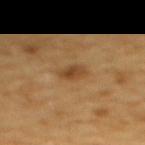patient: female, roughly 55 years of age | location: the upper back | acquisition: 15 mm crop, total-body photography | size: about 3 mm | image-analysis metrics: a lesion color around L≈45 a*≈20 b*≈37 in CIELAB, a lesion–skin lightness drop of about 10, and a normalized lesion–skin contrast near 7.5; a nevus-likeness score of about 30/100 and lesion-presence confidence of about 100/100.